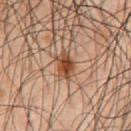No biopsy was performed on this lesion — it was imaged during a full skin examination and was not determined to be concerning. This is a cross-polarized tile. The lesion's longest dimension is about 3.5 mm. A male patient aged 58–62. On the chest. Cropped from a total-body skin-imaging series; the visible field is about 15 mm.